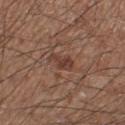Case summary:
* image-analysis metrics — roughly 8 lightness units darker than nearby skin and a normalized lesion–skin contrast near 7; a border-irregularity rating of about 4.5/10, a color-variation rating of about 1.5/10, and peripheral color asymmetry of about 0.5; lesion-presence confidence of about 100/100
* imaging modality — ~15 mm crop, total-body skin-cancer survey
* lighting — white-light
* body site — the leg
* diameter — about 3 mm
* patient — male, in their 60s